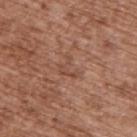workup — catalogued during a skin exam; not biopsied
location — the upper back
acquisition — ~15 mm crop, total-body skin-cancer survey
tile lighting — white-light illumination
patient — male, aged around 75
TBP lesion metrics — a lesion area of about 4.5 mm² and a shape-asymmetry score of about 0.7 (0 = symmetric); internal color variation of about 1 on a 0–10 scale and peripheral color asymmetry of about 0.5; a nevus-likeness score of about 0/100 and a detector confidence of about 100 out of 100 that the crop contains a lesion
size — ≈3 mm